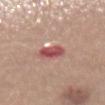  biopsy_status: not biopsied; imaged during a skin examination
  automated_metrics:
    border_irregularity_0_10: 2.0
    color_variation_0_10: 5.0
    peripheral_color_asymmetry: 1.5
    nevus_likeness_0_100: 0
    lesion_detection_confidence_0_100: 100
  patient:
    sex: female
    age_approx: 35
  site: abdomen
  image:
    source: total-body photography crop
    field_of_view_mm: 15
  lesion_size:
    long_diameter_mm_approx: 3.0